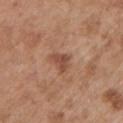Case summary:
• biopsy status · imaged on a skin check; not biopsied
• TBP lesion metrics · an area of roughly 4.5 mm²; a mean CIELAB color near L≈48 a*≈23 b*≈29 and about 10 CIELAB-L* units darker than the surrounding skin; an automated nevus-likeness rating near 55 out of 100 and a lesion-detection confidence of about 100/100
• location · the left upper arm
• lesion size · ≈3 mm
• image · 15 mm crop, total-body photography
• illumination · white-light
• patient · male, in their mid-50s